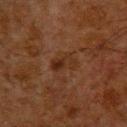Case summary:
* patient · male, in their 60s
* image · ~15 mm crop, total-body skin-cancer survey
* body site · the upper back